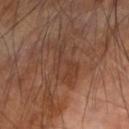workup = no biopsy performed (imaged during a skin exam)
body site = the arm
subject = male, aged 68–72
imaging modality = ~15 mm crop, total-body skin-cancer survey